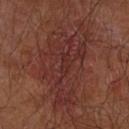Notes:
- follow-up: no biopsy performed (imaged during a skin exam)
- site: the arm
- illumination: cross-polarized
- acquisition: ~15 mm tile from a whole-body skin photo
- lesion diameter: ≈11 mm
- patient: male, in their mid-60s
- TBP lesion metrics: a lesion area of about 40 mm², a shape eccentricity near 0.85, and a shape-asymmetry score of about 0.45 (0 = symmetric); a mean CIELAB color near L≈30 a*≈23 b*≈22, roughly 6 lightness units darker than nearby skin, and a lesion-to-skin contrast of about 6.5 (normalized; higher = more distinct); a detector confidence of about 95 out of 100 that the crop contains a lesion